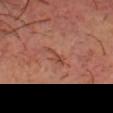| feature | finding |
|---|---|
| workup | imaged on a skin check; not biopsied |
| automated lesion analysis | a shape eccentricity near 0.9 and two-axis asymmetry of about 0.45; a border-irregularity index near 5.5/10, a color-variation rating of about 0.5/10, and a peripheral color-asymmetry measure near 0; an automated nevus-likeness rating near 0 out of 100 and lesion-presence confidence of about 75/100 |
| body site | the head or neck |
| acquisition | ~15 mm tile from a whole-body skin photo |
| illumination | cross-polarized illumination |
| patient | male, aged around 55 |